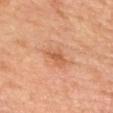Recorded during total-body skin imaging; not selected for excision or biopsy.